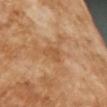TBP lesion metrics: a classifier nevus-likeness of about 0/100 and lesion-presence confidence of about 100/100
illumination: cross-polarized illumination
lesion size: ≈2.5 mm
image: ~15 mm tile from a whole-body skin photo
subject: male, aged 63–67